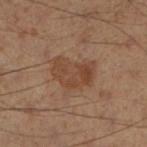No biopsy was performed on this lesion — it was imaged during a full skin examination and was not determined to be concerning. The tile uses cross-polarized illumination. From the right lower leg. A male patient in their 50s. Approximately 4.5 mm at its widest. A lesion tile, about 15 mm wide, cut from a 3D total-body photograph.